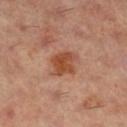Q: Was a biopsy performed?
A: imaged on a skin check; not biopsied
Q: What is the anatomic site?
A: the right lower leg
Q: What did automated image analysis measure?
A: a lesion area of about 7.5 mm², a shape eccentricity near 0.65, and two-axis asymmetry of about 0.25; a mean CIELAB color near L≈49 a*≈26 b*≈34, roughly 11 lightness units darker than nearby skin, and a lesion-to-skin contrast of about 9 (normalized; higher = more distinct); an automated nevus-likeness rating near 65 out of 100 and lesion-presence confidence of about 100/100
Q: How large is the lesion?
A: ≈3.5 mm
Q: How was the tile lit?
A: cross-polarized illumination
Q: What kind of image is this?
A: ~15 mm tile from a whole-body skin photo
Q: Who is the patient?
A: female, roughly 60 years of age